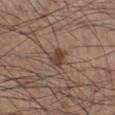notes=total-body-photography surveillance lesion; no biopsy | size=≈2.5 mm | acquisition=15 mm crop, total-body photography | tile lighting=white-light illumination | site=the left lower leg | subject=male, roughly 55 years of age.